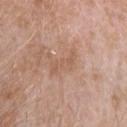Imaged during a routine full-body skin examination; the lesion was not biopsied and no histopathology is available.
Longest diameter approximately 4 mm.
A female patient about 25 years old.
A roughly 15 mm field-of-view crop from a total-body skin photograph.
Captured under white-light illumination.
The lesion is on the head or neck.
Automated tile analysis of the lesion measured a mean CIELAB color near L≈58 a*≈20 b*≈30 and a normalized lesion–skin contrast near 5. The analysis additionally found a border-irregularity index near 6/10, internal color variation of about 1 on a 0–10 scale, and a peripheral color-asymmetry measure near 0.